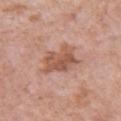Imaged during a routine full-body skin examination; the lesion was not biopsied and no histopathology is available.
Longest diameter approximately 4.5 mm.
A male subject aged around 55.
A roughly 15 mm field-of-view crop from a total-body skin photograph.
The lesion is located on the left upper arm.
The tile uses white-light illumination.
The total-body-photography lesion software estimated a shape eccentricity near 0.65 and two-axis asymmetry of about 0.25. It also reported an average lesion color of about L≈55 a*≈23 b*≈29 (CIELAB) and roughly 10 lightness units darker than nearby skin. It also reported a border-irregularity index near 3.5/10 and a within-lesion color-variation index near 4.5/10. The analysis additionally found an automated nevus-likeness rating near 15 out of 100 and a detector confidence of about 100 out of 100 that the crop contains a lesion.